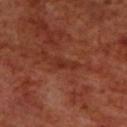This lesion was catalogued during total-body skin photography and was not selected for biopsy. The lesion is on the back. The subject is a male about 70 years old. This is a cross-polarized tile. The recorded lesion diameter is about 3 mm. Automated image analysis of the tile measured a footprint of about 3 mm² and a shape-asymmetry score of about 0.45 (0 = symmetric). It also reported a mean CIELAB color near L≈30 a*≈26 b*≈29 and a normalized border contrast of about 6. The software also gave border irregularity of about 5 on a 0–10 scale and a peripheral color-asymmetry measure near 0. The software also gave an automated nevus-likeness rating near 0 out of 100 and a lesion-detection confidence of about 65/100. A lesion tile, about 15 mm wide, cut from a 3D total-body photograph.